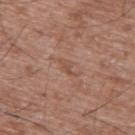Clinical impression:
The lesion was photographed on a routine skin check and not biopsied; there is no pathology result.
Context:
Automated image analysis of the tile measured a footprint of about 2.5 mm², an outline eccentricity of about 0.9 (0 = round, 1 = elongated), and a symmetry-axis asymmetry near 0.45. The software also gave border irregularity of about 5 on a 0–10 scale, internal color variation of about 0 on a 0–10 scale, and radial color variation of about 0. The subject is a male aged 48 to 52. This is a white-light tile. Longest diameter approximately 2.5 mm. A region of skin cropped from a whole-body photographic capture, roughly 15 mm wide. The lesion is located on the upper back.